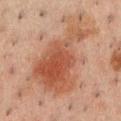The lesion was photographed on a routine skin check and not biopsied; there is no pathology result. Located on the chest. A male subject, approximately 50 years of age. A region of skin cropped from a whole-body photographic capture, roughly 15 mm wide. About 11 mm across. Automated image analysis of the tile measured a footprint of about 42 mm², an eccentricity of roughly 0.85, and two-axis asymmetry of about 0.5. The analysis additionally found border irregularity of about 8 on a 0–10 scale, a color-variation rating of about 5/10, and a peripheral color-asymmetry measure near 1.5. It also reported a classifier nevus-likeness of about 95/100.